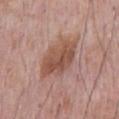Q: Is there a histopathology result?
A: catalogued during a skin exam; not biopsied
Q: How was this image acquired?
A: ~15 mm tile from a whole-body skin photo
Q: What are the patient's age and sex?
A: male, roughly 70 years of age
Q: Illumination type?
A: white-light illumination
Q: What is the lesion's diameter?
A: ≈6.5 mm
Q: Where on the body is the lesion?
A: the chest